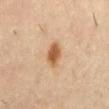Q: Was this lesion biopsied?
A: total-body-photography surveillance lesion; no biopsy
Q: Lesion location?
A: the mid back
Q: How was this image acquired?
A: total-body-photography crop, ~15 mm field of view
Q: Who is the patient?
A: male, in their mid-50s
Q: How was the tile lit?
A: cross-polarized
Q: What did automated image analysis measure?
A: an average lesion color of about L≈56 a*≈21 b*≈37 (CIELAB), about 13 CIELAB-L* units darker than the surrounding skin, and a lesion-to-skin contrast of about 9.5 (normalized; higher = more distinct); border irregularity of about 2 on a 0–10 scale; a classifier nevus-likeness of about 95/100